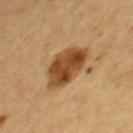This lesion was catalogued during total-body skin photography and was not selected for biopsy. On the upper back. This image is a 15 mm lesion crop taken from a total-body photograph. A female subject, aged 58 to 62. The tile uses cross-polarized illumination. Approximately 6 mm at its widest.